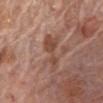  biopsy_status: not biopsied; imaged during a skin examination
  lighting: white-light
  site: chest
  lesion_size:
    long_diameter_mm_approx: 5.5
  image:
    source: total-body photography crop
    field_of_view_mm: 15
  patient:
    sex: male
    age_approx: 80